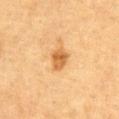The lesion was photographed on a routine skin check and not biopsied; there is no pathology result. The lesion is on the abdomen. The lesion-visualizer software estimated an area of roughly 4.5 mm² and a symmetry-axis asymmetry near 0.25. And it measured a border-irregularity rating of about 2.5/10, internal color variation of about 3.5 on a 0–10 scale, and a peripheral color-asymmetry measure near 1.5. A roughly 15 mm field-of-view crop from a total-body skin photograph. The subject is a male aged around 85 or older. Approximately 2.5 mm at its widest.